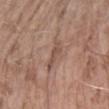Clinical summary:
Approximately 4 mm at its widest. A 15 mm close-up extracted from a 3D total-body photography capture. From the right forearm. A female subject aged around 75. Imaged with white-light lighting.